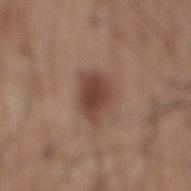follow-up: catalogued during a skin exam; not biopsied
automated metrics: a border-irregularity rating of about 2.5/10 and internal color variation of about 3.5 on a 0–10 scale; an automated nevus-likeness rating near 95 out of 100 and a lesion-detection confidence of about 100/100
lesion diameter: ≈4.5 mm
image source: ~15 mm tile from a whole-body skin photo
site: the mid back
subject: male, aged 58–62